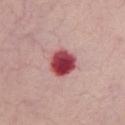<case>
  <biopsy_status>not biopsied; imaged during a skin examination</biopsy_status>
  <site>chest</site>
  <patient>
    <sex>female</sex>
    <age_approx>70</age_approx>
  </patient>
  <automated_metrics>
    <border_irregularity_0_10>1.5</border_irregularity_0_10>
    <color_variation_0_10>6.5</color_variation_0_10>
    <peripheral_color_asymmetry>2.0</peripheral_color_asymmetry>
  </automated_metrics>
  <lesion_size>
    <long_diameter_mm_approx>3.5</long_diameter_mm_approx>
  </lesion_size>
  <image>
    <source>total-body photography crop</source>
    <field_of_view_mm>15</field_of_view_mm>
  </image>
</case>